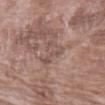• notes: no biopsy performed (imaged during a skin exam)
• tile lighting: white-light
• patient: female, aged 68–72
• diameter: ≈3.5 mm
• acquisition: ~15 mm tile from a whole-body skin photo
• body site: the left forearm